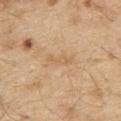The lesion was tiled from a total-body skin photograph and was not biopsied. A 15 mm crop from a total-body photograph taken for skin-cancer surveillance. The lesion is on the upper back. The lesion's longest dimension is about 3.5 mm. A male subject, aged 68 to 72. An algorithmic analysis of the crop reported a border-irregularity rating of about 5.5/10, a within-lesion color-variation index near 0/10, and peripheral color asymmetry of about 0. The tile uses white-light illumination.